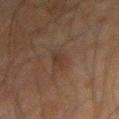Recorded during total-body skin imaging; not selected for excision or biopsy. Located on the arm. A male patient about 60 years old. Automated tile analysis of the lesion measured an average lesion color of about L≈26 a*≈13 b*≈19 (CIELAB), a lesion–skin lightness drop of about 4, and a lesion-to-skin contrast of about 5.5 (normalized; higher = more distinct). The analysis additionally found internal color variation of about 1 on a 0–10 scale and radial color variation of about 0.5. Cropped from a whole-body photographic skin survey; the tile spans about 15 mm. Measured at roughly 3.5 mm in maximum diameter. This is a cross-polarized tile.